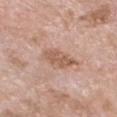Clinical impression: Part of a total-body skin-imaging series; this lesion was reviewed on a skin check and was not flagged for biopsy. Clinical summary: The subject is a female about 75 years old. Measured at roughly 4.5 mm in maximum diameter. Cropped from a whole-body photographic skin survey; the tile spans about 15 mm. Located on the chest.